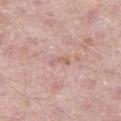Q: Is there a histopathology result?
A: no biopsy performed (imaged during a skin exam)